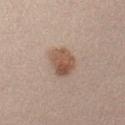Q: Is there a histopathology result?
A: no biopsy performed (imaged during a skin exam)
Q: How was this image acquired?
A: ~15 mm crop, total-body skin-cancer survey
Q: What lighting was used for the tile?
A: white-light illumination
Q: What are the patient's age and sex?
A: female, in their 30s
Q: What is the lesion's diameter?
A: about 4 mm
Q: Where on the body is the lesion?
A: the left upper arm